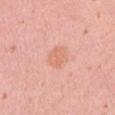No biopsy was performed on this lesion — it was imaged during a full skin examination and was not determined to be concerning. The recorded lesion diameter is about 3.5 mm. Cropped from a whole-body photographic skin survey; the tile spans about 15 mm. The lesion is on the right upper arm. A female subject, roughly 15 years of age.